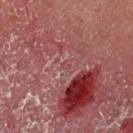Assessment: Recorded during total-body skin imaging; not selected for excision or biopsy. Clinical summary: Longest diameter approximately 1 mm. A male subject in their mid- to late 50s. The lesion is on the left upper arm. A roughly 15 mm field-of-view crop from a total-body skin photograph. This is a cross-polarized tile.